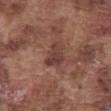Clinical summary: The subject is a male aged around 75. The lesion is on the abdomen. A 15 mm close-up extracted from a 3D total-body photography capture.